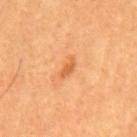<lesion>
<image>
  <source>total-body photography crop</source>
  <field_of_view_mm>15</field_of_view_mm>
</image>
<automated_metrics>
  <area_mm2_approx>3.0</area_mm2_approx>
  <eccentricity>0.9</eccentricity>
  <shape_asymmetry>0.4</shape_asymmetry>
  <vs_skin_darker_L>9.0</vs_skin_darker_L>
  <vs_skin_contrast_norm>6.5</vs_skin_contrast_norm>
  <border_irregularity_0_10>3.5</border_irregularity_0_10>
  <color_variation_0_10>0.5</color_variation_0_10>
  <peripheral_color_asymmetry>0.0</peripheral_color_asymmetry>
</automated_metrics>
<lesion_size>
  <long_diameter_mm_approx>2.5</long_diameter_mm_approx>
</lesion_size>
<lighting>cross-polarized</lighting>
<patient>
  <sex>male</sex>
  <age_approx>60</age_approx>
</patient>
<site>back</site>
</lesion>